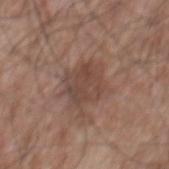Case summary:
• image source: ~15 mm tile from a whole-body skin photo
• subject: male, in their mid- to late 50s
• lesion size: about 4.5 mm
• image-analysis metrics: an eccentricity of roughly 0.6 and a shape-asymmetry score of about 0.2 (0 = symmetric); border irregularity of about 2.5 on a 0–10 scale, internal color variation of about 3 on a 0–10 scale, and a peripheral color-asymmetry measure near 1; a classifier nevus-likeness of about 0/100 and a lesion-detection confidence of about 100/100
• location: the mid back